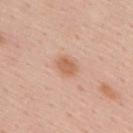A close-up tile cropped from a whole-body skin photograph, about 15 mm across. About 2.5 mm across. An algorithmic analysis of the crop reported a mean CIELAB color near L≈61 a*≈23 b*≈33 and a normalized lesion–skin contrast near 7. The software also gave a border-irregularity index near 2/10, a color-variation rating of about 2/10, and radial color variation of about 1. And it measured a lesion-detection confidence of about 100/100. This is a white-light tile. The lesion is on the back. A male patient roughly 55 years of age.